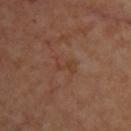The lesion was photographed on a routine skin check and not biopsied; there is no pathology result. About 3 mm across. Located on the upper back. A roughly 15 mm field-of-view crop from a total-body skin photograph. A female patient, approximately 45 years of age.